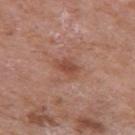The lesion was photographed on a routine skin check and not biopsied; there is no pathology result.
A male patient, aged approximately 70.
The lesion is on the right upper arm.
Cropped from a total-body skin-imaging series; the visible field is about 15 mm.
Captured under white-light illumination.
Automated image analysis of the tile measured a footprint of about 4.5 mm² and a symmetry-axis asymmetry near 0.3. The analysis additionally found a lesion-to-skin contrast of about 6.5 (normalized; higher = more distinct). It also reported a classifier nevus-likeness of about 25/100 and a detector confidence of about 100 out of 100 that the crop contains a lesion.
The lesion's longest dimension is about 3 mm.